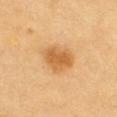notes = no biopsy performed (imaged during a skin exam) | subject = male, in their 60s | imaging modality = total-body-photography crop, ~15 mm field of view | location = the chest | TBP lesion metrics = a lesion area of about 11 mm² and an eccentricity of roughly 0.7; a mean CIELAB color near L≈61 a*≈22 b*≈45, about 11 CIELAB-L* units darker than the surrounding skin, and a normalized border contrast of about 7.5; a color-variation rating of about 3/10 and radial color variation of about 1 | lesion diameter = ~4 mm (longest diameter).